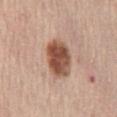– biopsy status · imaged on a skin check; not biopsied
– subject · female, aged around 65
– tile lighting · white-light illumination
– image · 15 mm crop, total-body photography
– size · about 5.5 mm
– site · the lower back